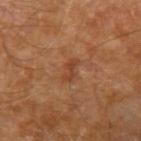Clinical impression: Part of a total-body skin-imaging series; this lesion was reviewed on a skin check and was not flagged for biopsy. Image and clinical context: The tile uses cross-polarized illumination. A lesion tile, about 15 mm wide, cut from a 3D total-body photograph. From the left upper arm. Automated image analysis of the tile measured a mean CIELAB color near L≈41 a*≈24 b*≈34 and a lesion–skin lightness drop of about 7. The analysis additionally found an automated nevus-likeness rating near 0 out of 100. A male patient, aged 68–72. The lesion's longest dimension is about 2.5 mm.